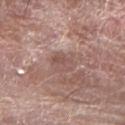Findings:
– imaging modality: ~15 mm tile from a whole-body skin photo
– body site: the left forearm
– patient: male, aged 73–77